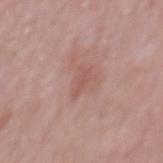Assessment:
The lesion was photographed on a routine skin check and not biopsied; there is no pathology result.
Background:
On the back. The recorded lesion diameter is about 3 mm. The patient is a male about 50 years old. This image is a 15 mm lesion crop taken from a total-body photograph. An algorithmic analysis of the crop reported border irregularity of about 4.5 on a 0–10 scale, a color-variation rating of about 0/10, and a peripheral color-asymmetry measure near 0. Imaged with white-light lighting.